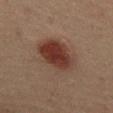No biopsy was performed on this lesion — it was imaged during a full skin examination and was not determined to be concerning. This image is a 15 mm lesion crop taken from a total-body photograph. The tile uses cross-polarized illumination. The lesion is located on the chest. The subject is a male approximately 45 years of age. Automated image analysis of the tile measured a lesion area of about 19 mm², an outline eccentricity of about 0.65 (0 = round, 1 = elongated), and a shape-asymmetry score of about 0.15 (0 = symmetric). The analysis additionally found a border-irregularity index near 1.5/10, a within-lesion color-variation index near 6/10, and radial color variation of about 2. It also reported a nevus-likeness score of about 100/100. Measured at roughly 5.5 mm in maximum diameter.